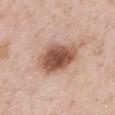Clinical summary: The lesion's longest dimension is about 5.5 mm. Located on the mid back. A male subject in their 50s. A close-up tile cropped from a whole-body skin photograph, about 15 mm across. Imaged with white-light lighting. Automated tile analysis of the lesion measured a border-irregularity index near 2.5/10, internal color variation of about 6 on a 0–10 scale, and radial color variation of about 2. The analysis additionally found a nevus-likeness score of about 95/100 and a detector confidence of about 100 out of 100 that the crop contains a lesion.